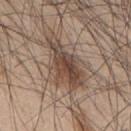Acquisition and patient details:
The lesion is located on the upper back. Cropped from a whole-body photographic skin survey; the tile spans about 15 mm. The subject is a male aged 43 to 47.